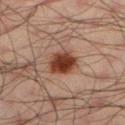{
  "biopsy_status": "not biopsied; imaged during a skin examination",
  "lighting": "cross-polarized",
  "site": "leg",
  "patient": {
    "sex": "male",
    "age_approx": 35
  },
  "lesion_size": {
    "long_diameter_mm_approx": 4.0
  },
  "image": {
    "source": "total-body photography crop",
    "field_of_view_mm": 15
  }
}